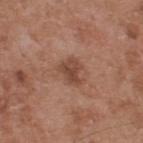Q: Was a biopsy performed?
A: total-body-photography surveillance lesion; no biopsy
Q: What kind of image is this?
A: ~15 mm crop, total-body skin-cancer survey
Q: How large is the lesion?
A: ~3 mm (longest diameter)
Q: Illumination type?
A: white-light
Q: What is the anatomic site?
A: the upper back
Q: Who is the patient?
A: male, aged approximately 55